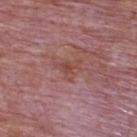This lesion was catalogued during total-body skin photography and was not selected for biopsy. From the upper back. The patient is a male aged around 75. Cropped from a total-body skin-imaging series; the visible field is about 15 mm. The tile uses white-light illumination. Measured at roughly 3 mm in maximum diameter.